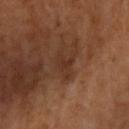  biopsy_status: not biopsied; imaged during a skin examination
  site: arm
  image:
    source: total-body photography crop
    field_of_view_mm: 15
  lighting: cross-polarized
  patient:
    sex: female
    age_approx: 65
  automated_metrics:
    area_mm2_approx: 4.5
    shape_asymmetry: 0.45
    cielab_L: 32
    cielab_a: 19
    cielab_b: 27
    vs_skin_darker_L: 6.0
    vs_skin_contrast_norm: 6.0
    border_irregularity_0_10: 6.0
    color_variation_0_10: 1.5
    peripheral_color_asymmetry: 0.5
  lesion_size:
    long_diameter_mm_approx: 4.0